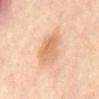| field | value |
|---|---|
| imaging modality | total-body-photography crop, ~15 mm field of view |
| subject | female, aged 48 to 52 |
| lesion size | ~3.5 mm (longest diameter) |
| lighting | cross-polarized |
| body site | the abdomen |